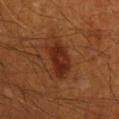Assessment:
Part of a total-body skin-imaging series; this lesion was reviewed on a skin check and was not flagged for biopsy.
Image and clinical context:
From the mid back. A close-up tile cropped from a whole-body skin photograph, about 15 mm across. A male patient about 60 years old. The total-body-photography lesion software estimated an automated nevus-likeness rating near 90 out of 100 and a lesion-detection confidence of about 100/100. Imaged with cross-polarized lighting.